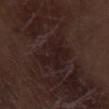Imaged with white-light lighting. The total-body-photography lesion software estimated a lesion area of about 14 mm² and a shape-asymmetry score of about 0.2 (0 = symmetric). And it measured an average lesion color of about L≈18 a*≈15 b*≈14 (CIELAB), about 5 CIELAB-L* units darker than the surrounding skin, and a normalized lesion–skin contrast near 6.5. And it measured a border-irregularity index near 2.5/10 and peripheral color asymmetry of about 1. Cropped from a whole-body photographic skin survey; the tile spans about 15 mm. A male patient, aged around 70. On the left thigh. About 5 mm across.